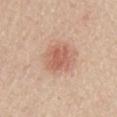• notes: no biopsy performed (imaged during a skin exam)
• location: the lower back
• image source: ~15 mm tile from a whole-body skin photo
• automated lesion analysis: a mean CIELAB color near L≈60 a*≈24 b*≈30 and a normalized lesion–skin contrast near 6.5; a color-variation rating of about 3/10 and peripheral color asymmetry of about 1; an automated nevus-likeness rating near 95 out of 100 and lesion-presence confidence of about 100/100
• subject: male, in their mid-60s